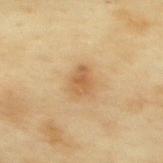Captured during whole-body skin photography for melanoma surveillance; the lesion was not biopsied. The patient is a female aged 58 to 62. A region of skin cropped from a whole-body photographic capture, roughly 15 mm wide. Located on the upper back. The recorded lesion diameter is about 3 mm.